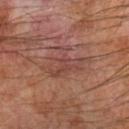Recorded during total-body skin imaging; not selected for excision or biopsy. A male subject aged approximately 70. The total-body-photography lesion software estimated a footprint of about 12 mm². It also reported about 6 CIELAB-L* units darker than the surrounding skin and a lesion-to-skin contrast of about 5.5 (normalized; higher = more distinct). Cropped from a whole-body photographic skin survey; the tile spans about 15 mm. From the left forearm. Longest diameter approximately 6 mm.